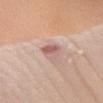{"biopsy_status": "not biopsied; imaged during a skin examination", "patient": {"sex": "male", "age_approx": 45}, "lighting": "white-light", "site": "left forearm", "image": {"source": "total-body photography crop", "field_of_view_mm": 15}, "lesion_size": {"long_diameter_mm_approx": 3.5}}